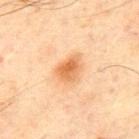Notes:
* notes · no biopsy performed (imaged during a skin exam)
* diameter · ≈3.5 mm
* image · ~15 mm tile from a whole-body skin photo
* tile lighting · cross-polarized illumination
* patient · male, aged 68–72
* body site · the chest